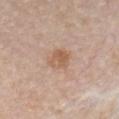The lesion was photographed on a routine skin check and not biopsied; there is no pathology result. The lesion is on the chest. Automated tile analysis of the lesion measured an area of roughly 6.5 mm², an eccentricity of roughly 0.5, and a symmetry-axis asymmetry near 0.15. The analysis additionally found a lesion–skin lightness drop of about 8. It also reported border irregularity of about 1.5 on a 0–10 scale, internal color variation of about 3.5 on a 0–10 scale, and radial color variation of about 1.5. The software also gave a nevus-likeness score of about 15/100 and a detector confidence of about 100 out of 100 that the crop contains a lesion. The subject is a female about 65 years old. The tile uses white-light illumination. A 15 mm crop from a total-body photograph taken for skin-cancer surveillance.